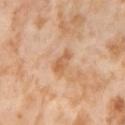Imaged during a routine full-body skin examination; the lesion was not biopsied and no histopathology is available. This image is a 15 mm lesion crop taken from a total-body photograph. The lesion is on the right thigh. Captured under cross-polarized illumination. A female subject, in their mid- to late 50s.